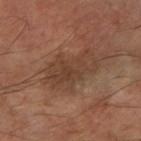Findings:
- follow-up: imaged on a skin check; not biopsied
- lighting: cross-polarized illumination
- body site: the right forearm
- automated lesion analysis: a mean CIELAB color near L≈40 a*≈18 b*≈28, about 7 CIELAB-L* units darker than the surrounding skin, and a normalized border contrast of about 6; a border-irregularity index near 4/10, a within-lesion color-variation index near 3.5/10, and peripheral color asymmetry of about 1.5; an automated nevus-likeness rating near 0 out of 100
- diameter: about 5.5 mm
- patient: male, about 55 years old
- acquisition: ~15 mm crop, total-body skin-cancer survey